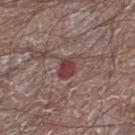Impression:
Part of a total-body skin-imaging series; this lesion was reviewed on a skin check and was not flagged for biopsy.
Image and clinical context:
The recorded lesion diameter is about 3.5 mm. The lesion is located on the right lower leg. An algorithmic analysis of the crop reported a footprint of about 5.5 mm², an eccentricity of roughly 0.75, and two-axis asymmetry of about 0.2. And it measured a nevus-likeness score of about 15/100. The tile uses white-light illumination. The subject is a male approximately 65 years of age. A 15 mm close-up extracted from a 3D total-body photography capture.